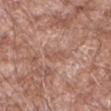The lesion was tiled from a total-body skin photograph and was not biopsied. Located on the left forearm. A male patient roughly 60 years of age. A roughly 15 mm field-of-view crop from a total-body skin photograph. Automated image analysis of the tile measured a lesion area of about 2.5 mm² and a shape-asymmetry score of about 0.35 (0 = symmetric). The analysis additionally found a lesion color around L≈54 a*≈21 b*≈28 in CIELAB and a normalized border contrast of about 5. Longest diameter approximately 2.5 mm. Imaged with white-light lighting.